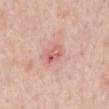patient — male, aged approximately 50
image — total-body-photography crop, ~15 mm field of view
location — the mid back
lesion diameter — ≈2.5 mm